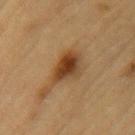Findings:
– notes — no biopsy performed (imaged during a skin exam)
– anatomic site — the left upper arm
– imaging modality — ~15 mm crop, total-body skin-cancer survey
– subject — male, in their mid- to late 80s or older
– lighting — cross-polarized illumination
– size — ~4 mm (longest diameter)